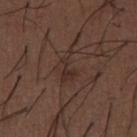Imaged during a routine full-body skin examination; the lesion was not biopsied and no histopathology is available. A 15 mm close-up tile from a total-body photography series done for melanoma screening. A male patient, aged 48–52. About 4 mm across. The lesion is on the abdomen. An algorithmic analysis of the crop reported a mean CIELAB color near L≈29 a*≈16 b*≈21, about 6 CIELAB-L* units darker than the surrounding skin, and a lesion-to-skin contrast of about 6 (normalized; higher = more distinct). The analysis additionally found an automated nevus-likeness rating near 0 out of 100 and a detector confidence of about 85 out of 100 that the crop contains a lesion. This is a white-light tile.